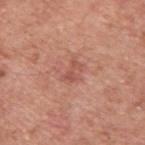Assessment:
Captured during whole-body skin photography for melanoma surveillance; the lesion was not biopsied.
Acquisition and patient details:
The lesion is on the upper back. The recorded lesion diameter is about 2.5 mm. Captured under white-light illumination. A close-up tile cropped from a whole-body skin photograph, about 15 mm across. A male subject, aged 53–57.